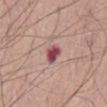• workup — no biopsy performed (imaged during a skin exam)